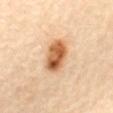Part of a total-body skin-imaging series; this lesion was reviewed on a skin check and was not flagged for biopsy. Located on the mid back. A female subject in their mid-60s. A 15 mm close-up tile from a total-body photography series done for melanoma screening. The lesion-visualizer software estimated an outline eccentricity of about 0.8 (0 = round, 1 = elongated). The analysis additionally found border irregularity of about 1.5 on a 0–10 scale, a within-lesion color-variation index near 8.5/10, and peripheral color asymmetry of about 3.